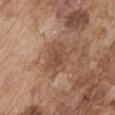The lesion was tiled from a total-body skin photograph and was not biopsied.
A male subject, approximately 75 years of age.
Located on the right upper arm.
This is a white-light tile.
Measured at roughly 4.5 mm in maximum diameter.
Automated image analysis of the tile measured a mean CIELAB color near L≈49 a*≈20 b*≈29, about 8 CIELAB-L* units darker than the surrounding skin, and a lesion-to-skin contrast of about 6 (normalized; higher = more distinct). And it measured a border-irregularity rating of about 2.5/10, a within-lesion color-variation index near 3.5/10, and peripheral color asymmetry of about 1. And it measured a classifier nevus-likeness of about 0/100.
A roughly 15 mm field-of-view crop from a total-body skin photograph.